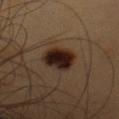workup: total-body-photography surveillance lesion; no biopsy | size: ~4.5 mm (longest diameter) | site: the left upper arm | automated lesion analysis: border irregularity of about 2 on a 0–10 scale and internal color variation of about 4.5 on a 0–10 scale; a nevus-likeness score of about 95/100 and a detector confidence of about 100 out of 100 that the crop contains a lesion | subject: male, roughly 60 years of age | imaging modality: 15 mm crop, total-body photography | tile lighting: cross-polarized illumination.